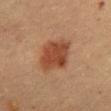Clinical impression: The lesion was tiled from a total-body skin photograph and was not biopsied. Background: A female patient roughly 60 years of age. A close-up tile cropped from a whole-body skin photograph, about 15 mm across. The lesion-visualizer software estimated a lesion color around L≈41 a*≈22 b*≈30 in CIELAB, roughly 10 lightness units darker than nearby skin, and a normalized lesion–skin contrast near 8.5. The analysis additionally found a lesion-detection confidence of about 100/100. The lesion is located on the chest. Approximately 5 mm at its widest. Imaged with cross-polarized lighting.